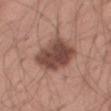notes — total-body-photography surveillance lesion; no biopsy
image-analysis metrics — border irregularity of about 2 on a 0–10 scale, internal color variation of about 4.5 on a 0–10 scale, and peripheral color asymmetry of about 1.5
subject — male, about 40 years old
lesion diameter — about 5.5 mm
imaging modality — total-body-photography crop, ~15 mm field of view
tile lighting — white-light
location — the right thigh